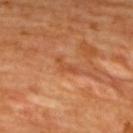notes=catalogued during a skin exam; not biopsied | subject=female, in their mid-40s | illumination=cross-polarized | anatomic site=the upper back | acquisition=15 mm crop, total-body photography | lesion diameter=~3 mm (longest diameter) | TBP lesion metrics=an average lesion color of about L≈50 a*≈28 b*≈39 (CIELAB), about 7 CIELAB-L* units darker than the surrounding skin, and a normalized lesion–skin contrast near 5.5; border irregularity of about 6.5 on a 0–10 scale, a color-variation rating of about 0/10, and peripheral color asymmetry of about 0; a nevus-likeness score of about 0/100 and a detector confidence of about 95 out of 100 that the crop contains a lesion.